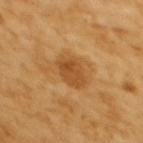Part of a total-body skin-imaging series; this lesion was reviewed on a skin check and was not flagged for biopsy.
A female patient in their mid-50s.
Imaged with cross-polarized lighting.
A lesion tile, about 15 mm wide, cut from a 3D total-body photograph.
Approximately 3.5 mm at its widest.
Located on the upper back.
The total-body-photography lesion software estimated an area of roughly 8 mm², an eccentricity of roughly 0.6, and two-axis asymmetry of about 0.15. The software also gave an average lesion color of about L≈50 a*≈24 b*≈43 (CIELAB), a lesion–skin lightness drop of about 10, and a lesion-to-skin contrast of about 7 (normalized; higher = more distinct). And it measured a nevus-likeness score of about 85/100 and lesion-presence confidence of about 100/100.